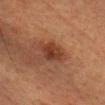A 15 mm close-up tile from a total-body photography series done for melanoma screening. The lesion is located on the head or neck. Imaged with cross-polarized lighting. A female subject aged 53 to 57. An algorithmic analysis of the crop reported a footprint of about 5.5 mm² and two-axis asymmetry of about 0.25. The analysis additionally found border irregularity of about 2.5 on a 0–10 scale, a color-variation rating of about 3/10, and peripheral color asymmetry of about 1.